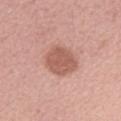{"biopsy_status": "not biopsied; imaged during a skin examination", "lighting": "white-light", "patient": {"sex": "female", "age_approx": 50}, "automated_metrics": {"border_irregularity_0_10": 1.5, "color_variation_0_10": 2.0, "peripheral_color_asymmetry": 0.5}, "site": "left forearm", "image": {"source": "total-body photography crop", "field_of_view_mm": 15}, "lesion_size": {"long_diameter_mm_approx": 4.0}}